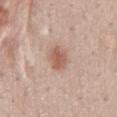Clinical impression:
No biopsy was performed on this lesion — it was imaged during a full skin examination and was not determined to be concerning.
Context:
Cropped from a total-body skin-imaging series; the visible field is about 15 mm. The total-body-photography lesion software estimated a lesion area of about 6 mm², an outline eccentricity of about 0.5 (0 = round, 1 = elongated), and a symmetry-axis asymmetry near 0.2. And it measured a border-irregularity index near 1.5/10, internal color variation of about 2.5 on a 0–10 scale, and radial color variation of about 1. Located on the mid back. This is a white-light tile. The subject is a female about 50 years old.